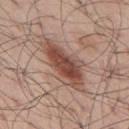Part of a total-body skin-imaging series; this lesion was reviewed on a skin check and was not flagged for biopsy. The lesion is located on the mid back. A male patient, in their mid-50s. The lesion's longest dimension is about 8 mm. A 15 mm crop from a total-body photograph taken for skin-cancer surveillance. Automated tile analysis of the lesion measured a mean CIELAB color near L≈50 a*≈20 b*≈26. The tile uses white-light illumination.